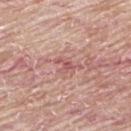Findings:
* notes — no biopsy performed (imaged during a skin exam)
* lesion size — ~2.5 mm (longest diameter)
* patient — male, in their mid-60s
* acquisition — ~15 mm crop, total-body skin-cancer survey
* tile lighting — white-light
* anatomic site — the back